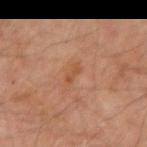Case summary:
- follow-up · total-body-photography surveillance lesion; no biopsy